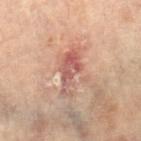notes = total-body-photography surveillance lesion; no biopsy.